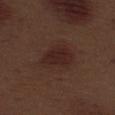Q: Is there a histopathology result?
A: no biopsy performed (imaged during a skin exam)
Q: Who is the patient?
A: male, aged 68–72
Q: What is the imaging modality?
A: 15 mm crop, total-body photography
Q: Automated lesion metrics?
A: a lesion area of about 7.5 mm² and an outline eccentricity of about 0.75 (0 = round, 1 = elongated); a border-irregularity rating of about 2/10 and a peripheral color-asymmetry measure near 0.5; a lesion-detection confidence of about 100/100
Q: Where on the body is the lesion?
A: the left thigh
Q: How was the tile lit?
A: white-light